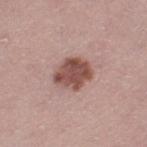This lesion was catalogued during total-body skin photography and was not selected for biopsy.
This is a white-light tile.
A female patient roughly 40 years of age.
A 15 mm close-up extracted from a 3D total-body photography capture.
The recorded lesion diameter is about 4 mm.
The lesion is located on the left thigh.